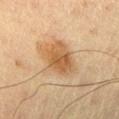biopsy status — no biopsy performed (imaged during a skin exam)
lighting — cross-polarized illumination
acquisition — 15 mm crop, total-body photography
patient — male, in their mid-60s
location — the chest
image-analysis metrics — a lesion area of about 16 mm² and a symmetry-axis asymmetry near 0.2; a lesion color around L≈53 a*≈17 b*≈35 in CIELAB, a lesion–skin lightness drop of about 9, and a normalized border contrast of about 7.5